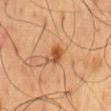Part of a total-body skin-imaging series; this lesion was reviewed on a skin check and was not flagged for biopsy.
This image is a 15 mm lesion crop taken from a total-body photograph.
A male patient, about 60 years old.
Approximately 2.5 mm at its widest.
Located on the mid back.
Imaged with cross-polarized lighting.
The total-body-photography lesion software estimated a mean CIELAB color near L≈40 a*≈20 b*≈32, roughly 10 lightness units darker than nearby skin, and a normalized lesion–skin contrast near 8.5. And it measured an automated nevus-likeness rating near 90 out of 100 and lesion-presence confidence of about 100/100.